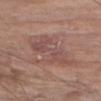<record>
  <biopsy_status>not biopsied; imaged during a skin examination</biopsy_status>
  <patient>
    <sex>male</sex>
    <age_approx>80</age_approx>
  </patient>
  <image>
    <source>total-body photography crop</source>
    <field_of_view_mm>15</field_of_view_mm>
  </image>
  <site>right upper arm</site>
</record>